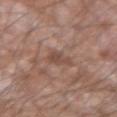This lesion was catalogued during total-body skin photography and was not selected for biopsy. Located on the left forearm. The subject is a male aged around 55. Longest diameter approximately 2.5 mm. Cropped from a whole-body photographic skin survey; the tile spans about 15 mm. The lesion-visualizer software estimated a nevus-likeness score of about 0/100 and a detector confidence of about 100 out of 100 that the crop contains a lesion.